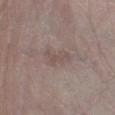Captured during whole-body skin photography for melanoma surveillance; the lesion was not biopsied. The tile uses white-light illumination. The lesion is located on the left lower leg. The patient is a male aged 58–62. This image is a 15 mm lesion crop taken from a total-body photograph.